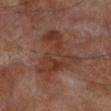biopsy status: no biopsy performed (imaged during a skin exam); imaging modality: ~15 mm crop, total-body skin-cancer survey; illumination: cross-polarized illumination; location: the left lower leg; patient: male, aged 68 to 72; lesion size: about 8 mm.